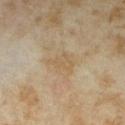This lesion was catalogued during total-body skin photography and was not selected for biopsy.
The subject is a female aged around 35.
Cropped from a whole-body photographic skin survey; the tile spans about 15 mm.
From the right upper arm.
Measured at roughly 3.5 mm in maximum diameter.
An algorithmic analysis of the crop reported an average lesion color of about L≈56 a*≈12 b*≈33 (CIELAB) and a normalized lesion–skin contrast near 4.5. It also reported a detector confidence of about 100 out of 100 that the crop contains a lesion.
Imaged with cross-polarized lighting.